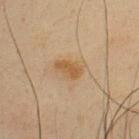Notes:
* body site · the back
* lighting · cross-polarized illumination
* automated metrics · a footprint of about 5 mm², a shape eccentricity near 0.85, and a symmetry-axis asymmetry near 0.25; border irregularity of about 2.5 on a 0–10 scale and internal color variation of about 2.5 on a 0–10 scale; a classifier nevus-likeness of about 65/100 and lesion-presence confidence of about 100/100
* diameter · ~3 mm (longest diameter)
* subject · male, approximately 35 years of age
* imaging modality · 15 mm crop, total-body photography